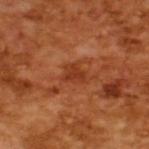Imaged during a routine full-body skin examination; the lesion was not biopsied and no histopathology is available. About 3 mm across. The subject is a male aged 63 to 67. This is a cross-polarized tile. The lesion-visualizer software estimated a lesion–skin lightness drop of about 8 and a normalized lesion–skin contrast near 6.5. This image is a 15 mm lesion crop taken from a total-body photograph.